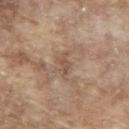| feature | finding |
|---|---|
| workup | catalogued during a skin exam; not biopsied |
| acquisition | ~15 mm tile from a whole-body skin photo |
| lighting | white-light illumination |
| diameter | ≈3 mm |
| patient | male, aged 63–67 |
| site | the upper back |